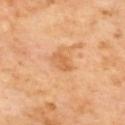Findings:
• notes — total-body-photography surveillance lesion; no biopsy
• image — 15 mm crop, total-body photography
• location — the upper back